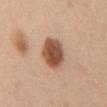Captured during whole-body skin photography for melanoma surveillance; the lesion was not biopsied.
Captured under white-light illumination.
From the chest.
About 4.5 mm across.
A female patient, aged 23 to 27.
A 15 mm close-up extracted from a 3D total-body photography capture.